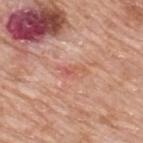workup: catalogued during a skin exam; not biopsied | location: the upper back | image: ~15 mm tile from a whole-body skin photo | illumination: white-light | subject: male, aged 78–82.